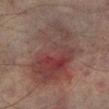<tbp_lesion>
  <biopsy_status>not biopsied; imaged during a skin examination</biopsy_status>
  <automated_metrics>
    <vs_skin_darker_L>8.0</vs_skin_darker_L>
    <vs_skin_contrast_norm>7.5</vs_skin_contrast_norm>
    <color_variation_0_10>9.0</color_variation_0_10>
    <peripheral_color_asymmetry>3.5</peripheral_color_asymmetry>
  </automated_metrics>
  <image>
    <source>total-body photography crop</source>
    <field_of_view_mm>15</field_of_view_mm>
  </image>
  <lesion_size>
    <long_diameter_mm_approx>11.0</long_diameter_mm_approx>
  </lesion_size>
  <lighting>cross-polarized</lighting>
  <patient>
    <sex>male</sex>
    <age_approx>75</age_approx>
  </patient>
  <site>left lower leg</site>
</tbp_lesion>